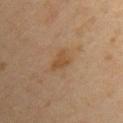{
  "patient": {
    "sex": "female",
    "age_approx": 45
  },
  "lighting": "cross-polarized",
  "lesion_size": {
    "long_diameter_mm_approx": 3.0
  },
  "image": {
    "source": "total-body photography crop",
    "field_of_view_mm": 15
  },
  "automated_metrics": {
    "area_mm2_approx": 4.0,
    "eccentricity": 0.75,
    "shape_asymmetry": 0.35,
    "vs_skin_contrast_norm": 6.5,
    "lesion_detection_confidence_0_100": 100
  },
  "site": "chest"
}